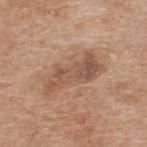Notes:
• follow-up: total-body-photography surveillance lesion; no biopsy
• tile lighting: white-light
• image source: ~15 mm tile from a whole-body skin photo
• location: the upper back
• patient: male, aged approximately 60